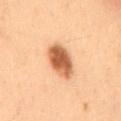A 15 mm close-up tile from a total-body photography series done for melanoma screening. A male patient, aged approximately 55. The lesion is located on the mid back.